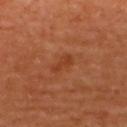biopsy status — no biopsy performed (imaged during a skin exam) | body site — the upper back | size — ~2.5 mm (longest diameter) | illumination — cross-polarized illumination | image — 15 mm crop, total-body photography | patient — female, aged 43–47 | automated metrics — an area of roughly 3.5 mm²; an automated nevus-likeness rating near 10 out of 100.